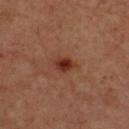biopsy_status: not biopsied; imaged during a skin examination
automated_metrics:
  area_mm2_approx: 4.0
  eccentricity: 0.65
  shape_asymmetry: 0.15
  vs_skin_darker_L: 11.0
  nevus_likeness_0_100: 95
image:
  source: total-body photography crop
  field_of_view_mm: 15
site: upper back
patient:
  sex: male
  age_approx: 65
lighting: cross-polarized